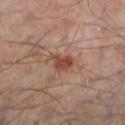{"patient": {"sex": "male", "age_approx": 55}, "image": {"source": "total-body photography crop", "field_of_view_mm": 15}, "site": "left lower leg"}